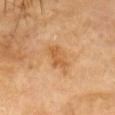- location — the head or neck
- lesion size — ≈4 mm
- tile lighting — cross-polarized
- patient — female, aged 68–72
- acquisition — ~15 mm crop, total-body skin-cancer survey
- automated metrics — an area of roughly 6.5 mm², an outline eccentricity of about 0.85 (0 = round, 1 = elongated), and a shape-asymmetry score of about 0.25 (0 = symmetric); a lesion color around L≈61 a*≈23 b*≈44 in CIELAB, about 9 CIELAB-L* units darker than the surrounding skin, and a normalized lesion–skin contrast near 6.5; a border-irregularity index near 3/10, internal color variation of about 2.5 on a 0–10 scale, and a peripheral color-asymmetry measure near 0.5